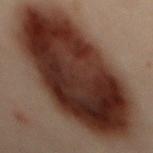Case summary:
– biopsy status — catalogued during a skin exam; not biopsied
– patient — male, about 50 years old
– lighting — cross-polarized illumination
– diameter — ≈17.5 mm
– body site — the back
– image — 15 mm crop, total-body photography
– image-analysis metrics — a lesion area of about 105 mm², a shape eccentricity near 0.9, and two-axis asymmetry of about 0.1; an average lesion color of about L≈25 a*≈16 b*≈20 (CIELAB), a lesion–skin lightness drop of about 16, and a lesion-to-skin contrast of about 16 (normalized; higher = more distinct); border irregularity of about 2 on a 0–10 scale and a color-variation rating of about 9/10